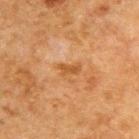Acquisition and patient details:
A region of skin cropped from a whole-body photographic capture, roughly 15 mm wide. The patient is a male aged around 50. On the upper back.